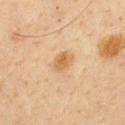Recorded during total-body skin imaging; not selected for excision or biopsy. This is a cross-polarized tile. The recorded lesion diameter is about 2.5 mm. Cropped from a total-body skin-imaging series; the visible field is about 15 mm. Located on the chest. The patient is a male aged around 60.